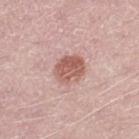<tbp_lesion>
  <biopsy_status>not biopsied; imaged during a skin examination</biopsy_status>
  <site>leg</site>
  <automated_metrics>
    <area_mm2_approx>9.5</area_mm2_approx>
    <eccentricity>0.55</eccentricity>
    <shape_asymmetry>0.1</shape_asymmetry>
    <vs_skin_darker_L>12.0</vs_skin_darker_L>
    <color_variation_0_10>4.0</color_variation_0_10>
    <peripheral_color_asymmetry>1.5</peripheral_color_asymmetry>
    <nevus_likeness_0_100>60</nevus_likeness_0_100>
    <lesion_detection_confidence_0_100>100</lesion_detection_confidence_0_100>
  </automated_metrics>
  <image>
    <source>total-body photography crop</source>
    <field_of_view_mm>15</field_of_view_mm>
  </image>
  <patient>
    <sex>male</sex>
    <age_approx>50</age_approx>
  </patient>
</tbp_lesion>